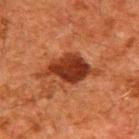* workup — catalogued during a skin exam; not biopsied
* lighting — cross-polarized
* acquisition — ~15 mm tile from a whole-body skin photo
* site — the upper back
* size — ~7 mm (longest diameter)
* subject — male, in their 60s